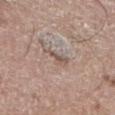Located on the left lower leg.
The lesion's longest dimension is about 3.5 mm.
A male patient, approximately 65 years of age.
The total-body-photography lesion software estimated a mean CIELAB color near L≈54 a*≈15 b*≈22, a lesion–skin lightness drop of about 9, and a normalized lesion–skin contrast near 6. The software also gave a border-irregularity rating of about 5.5/10, a within-lesion color-variation index near 2.5/10, and peripheral color asymmetry of about 1. It also reported a classifier nevus-likeness of about 0/100 and a detector confidence of about 75 out of 100 that the crop contains a lesion.
The tile uses white-light illumination.
A 15 mm crop from a total-body photograph taken for skin-cancer surveillance.